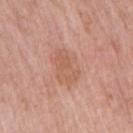Captured during whole-body skin photography for melanoma surveillance; the lesion was not biopsied.
A male subject, in their 60s.
A 15 mm close-up tile from a total-body photography series done for melanoma screening.
The lesion is on the right upper arm.